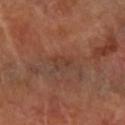Imaged during a routine full-body skin examination; the lesion was not biopsied and no histopathology is available.
The total-body-photography lesion software estimated an average lesion color of about L≈39 a*≈21 b*≈28 (CIELAB) and a lesion-to-skin contrast of about 4.5 (normalized; higher = more distinct).
Longest diameter approximately 2.5 mm.
A 15 mm crop from a total-body photograph taken for skin-cancer surveillance.
A female patient, about 60 years old.
The lesion is located on the left arm.